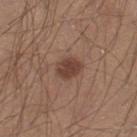Impression:
Recorded during total-body skin imaging; not selected for excision or biopsy.
Image and clinical context:
A roughly 15 mm field-of-view crop from a total-body skin photograph. Located on the right lower leg. A male subject in their 30s. An algorithmic analysis of the crop reported a footprint of about 6 mm², an outline eccentricity of about 0.65 (0 = round, 1 = elongated), and a shape-asymmetry score of about 0.15 (0 = symmetric). The software also gave a lesion color around L≈42 a*≈19 b*≈25 in CIELAB and a normalized border contrast of about 8.5. The software also gave a nevus-likeness score of about 90/100 and a detector confidence of about 100 out of 100 that the crop contains a lesion. The tile uses white-light illumination. Longest diameter approximately 3 mm.